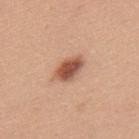• biopsy status · catalogued during a skin exam; not biopsied
• illumination · white-light illumination
• location · the upper back
• automated lesion analysis · a footprint of about 7 mm², a shape eccentricity near 0.8, and two-axis asymmetry of about 0.2; an average lesion color of about L≈54 a*≈24 b*≈31 (CIELAB) and about 15 CIELAB-L* units darker than the surrounding skin; a nevus-likeness score of about 100/100 and a detector confidence of about 100 out of 100 that the crop contains a lesion
• subject · female, aged 43–47
• image source · 15 mm crop, total-body photography